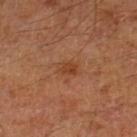{
  "biopsy_status": "not biopsied; imaged during a skin examination",
  "patient": {
    "sex": "male",
    "age_approx": 60
  },
  "image": {
    "source": "total-body photography crop",
    "field_of_view_mm": 15
  },
  "site": "right leg",
  "lesion_size": {
    "long_diameter_mm_approx": 2.5
  }
}